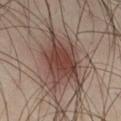Clinical impression: Part of a total-body skin-imaging series; this lesion was reviewed on a skin check and was not flagged for biopsy. Image and clinical context: Imaged with cross-polarized lighting. A male subject, about 40 years old. This image is a 15 mm lesion crop taken from a total-body photograph. From the right thigh.